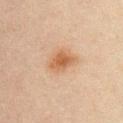  biopsy_status: not biopsied; imaged during a skin examination
  image:
    source: total-body photography crop
    field_of_view_mm: 15
  patient:
    sex: male
    age_approx: 30
  site: chest